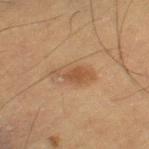<lesion>
  <biopsy_status>not biopsied; imaged during a skin examination</biopsy_status>
  <image>
    <source>total-body photography crop</source>
    <field_of_view_mm>15</field_of_view_mm>
  </image>
  <patient>
    <sex>male</sex>
    <age_approx>60</age_approx>
  </patient>
  <site>left thigh</site>
</lesion>